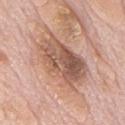<tbp_lesion>
  <image>
    <source>total-body photography crop</source>
    <field_of_view_mm>15</field_of_view_mm>
  </image>
  <lesion_size>
    <long_diameter_mm_approx>8.0</long_diameter_mm_approx>
  </lesion_size>
  <site>mid back</site>
  <patient>
    <sex>male</sex>
    <age_approx>75</age_approx>
  </patient>
  <automated_metrics>
    <area_mm2_approx>26.0</area_mm2_approx>
    <eccentricity>0.85</eccentricity>
    <cielab_L>57</cielab_L>
    <cielab_a>20</cielab_a>
    <cielab_b>28</cielab_b>
    <vs_skin_darker_L>13.0</vs_skin_darker_L>
    <vs_skin_contrast_norm>8.5</vs_skin_contrast_norm>
    <border_irregularity_0_10>4.0</border_irregularity_0_10>
    <peripheral_color_asymmetry>2.5</peripheral_color_asymmetry>
  </automated_metrics>
</tbp_lesion>